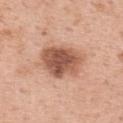Part of a total-body skin-imaging series; this lesion was reviewed on a skin check and was not flagged for biopsy.
Captured under white-light illumination.
From the back.
This image is a 15 mm lesion crop taken from a total-body photograph.
Approximately 5.5 mm at its widest.
An algorithmic analysis of the crop reported a footprint of about 18 mm², an outline eccentricity of about 0.7 (0 = round, 1 = elongated), and a shape-asymmetry score of about 0.2 (0 = symmetric). The software also gave a lesion color around L≈55 a*≈23 b*≈31 in CIELAB, a lesion–skin lightness drop of about 15, and a normalized lesion–skin contrast near 9.5. The software also gave internal color variation of about 5.5 on a 0–10 scale and radial color variation of about 1.5.
A female patient about 50 years old.